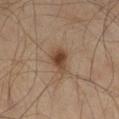Impression: Part of a total-body skin-imaging series; this lesion was reviewed on a skin check and was not flagged for biopsy. Image and clinical context: Measured at roughly 3 mm in maximum diameter. A lesion tile, about 15 mm wide, cut from a 3D total-body photograph. Automated image analysis of the tile measured an area of roughly 5.5 mm² and a shape-asymmetry score of about 0.35 (0 = symmetric). The software also gave a mean CIELAB color near L≈39 a*≈16 b*≈27, roughly 10 lightness units darker than nearby skin, and a normalized lesion–skin contrast near 9. On the right thigh. The subject is a male aged around 60.